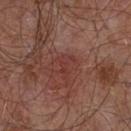Recorded during total-body skin imaging; not selected for excision or biopsy. A region of skin cropped from a whole-body photographic capture, roughly 15 mm wide. The lesion's longest dimension is about 3 mm. The patient is a male aged 53 to 57. The lesion is on the front of the torso. Imaged with white-light lighting. An algorithmic analysis of the crop reported a footprint of about 3 mm², a shape eccentricity near 0.85, and a shape-asymmetry score of about 0.45 (0 = symmetric). The analysis additionally found a within-lesion color-variation index near 0/10 and peripheral color asymmetry of about 0. The software also gave a nevus-likeness score of about 10/100 and a detector confidence of about 100 out of 100 that the crop contains a lesion.